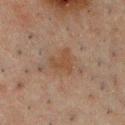Imaged during a routine full-body skin examination; the lesion was not biopsied and no histopathology is available. Imaged with cross-polarized lighting. The lesion is located on the chest. A 15 mm close-up extracted from a 3D total-body photography capture. Longest diameter approximately 3.5 mm. A male patient, in their 50s.